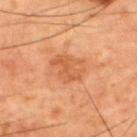The lesion was tiled from a total-body skin photograph and was not biopsied.
Captured under cross-polarized illumination.
Cropped from a whole-body photographic skin survey; the tile spans about 15 mm.
The recorded lesion diameter is about 3.5 mm.
On the upper back.
The subject is a male aged around 60.